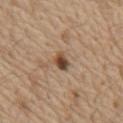Findings:
* follow-up · total-body-photography surveillance lesion; no biopsy
* lesion size · ~2.5 mm (longest diameter)
* patient · male, in their 70s
* anatomic site · the chest
* image source · ~15 mm tile from a whole-body skin photo